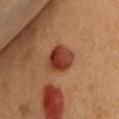Clinical impression:
The lesion was photographed on a routine skin check and not biopsied; there is no pathology result.
Acquisition and patient details:
A lesion tile, about 15 mm wide, cut from a 3D total-body photograph. A female patient, in their 40s. This is a cross-polarized tile. An algorithmic analysis of the crop reported a nevus-likeness score of about 100/100 and lesion-presence confidence of about 100/100. About 3.5 mm across. Located on the chest.